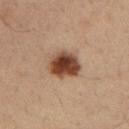A region of skin cropped from a whole-body photographic capture, roughly 15 mm wide. This is a cross-polarized tile. A male subject aged 33 to 37. Located on the right upper arm.